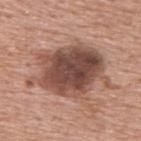The lesion was photographed on a routine skin check and not biopsied; there is no pathology result. The tile uses white-light illumination. The recorded lesion diameter is about 9 mm. From the upper back. A roughly 15 mm field-of-view crop from a total-body skin photograph. A male subject in their mid-70s.